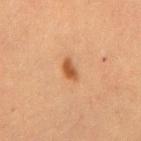A lesion tile, about 15 mm wide, cut from a 3D total-body photograph.
About 2.5 mm across.
The lesion is located on the mid back.
A male patient aged 48–52.
Imaged with cross-polarized lighting.